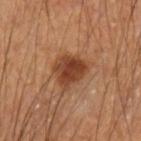The lesion was photographed on a routine skin check and not biopsied; there is no pathology result. A region of skin cropped from a whole-body photographic capture, roughly 15 mm wide. A male patient, in their mid- to late 60s. Located on the left forearm. The lesion-visualizer software estimated two-axis asymmetry of about 0.3. The software also gave an average lesion color of about L≈42 a*≈24 b*≈33 (CIELAB). And it measured a color-variation rating of about 4.5/10 and peripheral color asymmetry of about 1.5. And it measured a classifier nevus-likeness of about 95/100 and lesion-presence confidence of about 100/100.